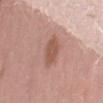This lesion was catalogued during total-body skin photography and was not selected for biopsy. A female patient aged 23 to 27. Captured under white-light illumination. Approximately 3.5 mm at its widest. This image is a 15 mm lesion crop taken from a total-body photograph.